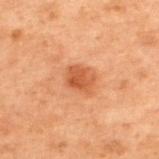Captured during whole-body skin photography for melanoma surveillance; the lesion was not biopsied.
About 2.5 mm across.
Located on the upper back.
Automated tile analysis of the lesion measured a lesion area of about 5.5 mm² and two-axis asymmetry of about 0.25. The analysis additionally found border irregularity of about 2.5 on a 0–10 scale, a within-lesion color-variation index near 2/10, and radial color variation of about 0.5.
A close-up tile cropped from a whole-body skin photograph, about 15 mm across.
This is a cross-polarized tile.
The patient is a male in their 70s.